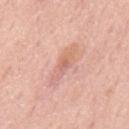workup = catalogued during a skin exam; not biopsied
acquisition = total-body-photography crop, ~15 mm field of view
location = the back
subject = male, aged around 75
lesion size = about 5.5 mm